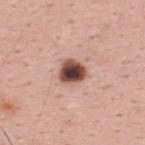notes: imaged on a skin check; not biopsied | automated metrics: a lesion area of about 7 mm², an outline eccentricity of about 0.5 (0 = round, 1 = elongated), and a shape-asymmetry score of about 0.2 (0 = symmetric); a nevus-likeness score of about 90/100 | lighting: white-light | subject: male, approximately 30 years of age | acquisition: ~15 mm crop, total-body skin-cancer survey | site: the upper back.